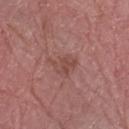No biopsy was performed on this lesion — it was imaged during a full skin examination and was not determined to be concerning.
Automated tile analysis of the lesion measured a footprint of about 5 mm² and an outline eccentricity of about 0.7 (0 = round, 1 = elongated). The software also gave roughly 7 lightness units darker than nearby skin and a lesion-to-skin contrast of about 5.5 (normalized; higher = more distinct). It also reported a classifier nevus-likeness of about 0/100 and a detector confidence of about 100 out of 100 that the crop contains a lesion.
A 15 mm close-up extracted from a 3D total-body photography capture.
Imaged with white-light lighting.
A male subject about 70 years old.
Approximately 3.5 mm at its widest.
Located on the left forearm.